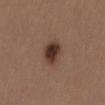<lesion>
  <patient>
    <sex>female</sex>
    <age_approx>40</age_approx>
  </patient>
  <site>left thigh</site>
  <image>
    <source>total-body photography crop</source>
    <field_of_view_mm>15</field_of_view_mm>
  </image>
</lesion>